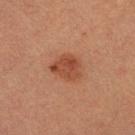workup: imaged on a skin check; not biopsied | patient: female, in their 40s | acquisition: ~15 mm crop, total-body skin-cancer survey | site: the right lower leg.